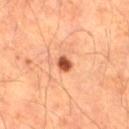Imaged during a routine full-body skin examination; the lesion was not biopsied and no histopathology is available.
A male subject, roughly 65 years of age.
A lesion tile, about 15 mm wide, cut from a 3D total-body photograph.
The lesion is located on the right thigh.
About 2 mm across.